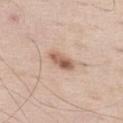Part of a total-body skin-imaging series; this lesion was reviewed on a skin check and was not flagged for biopsy. Imaged with white-light lighting. A region of skin cropped from a whole-body photographic capture, roughly 15 mm wide. On the abdomen. A male patient, aged around 55. The recorded lesion diameter is about 3.5 mm.